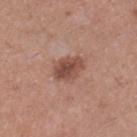Q: Was this lesion biopsied?
A: total-body-photography surveillance lesion; no biopsy
Q: Lesion size?
A: ≈3.5 mm
Q: Automated lesion metrics?
A: a lesion color around L≈48 a*≈22 b*≈26 in CIELAB, about 12 CIELAB-L* units darker than the surrounding skin, and a normalized lesion–skin contrast near 8.5
Q: What are the patient's age and sex?
A: female, aged approximately 30
Q: How was this image acquired?
A: ~15 mm crop, total-body skin-cancer survey
Q: Where on the body is the lesion?
A: the right lower leg
Q: Illumination type?
A: white-light